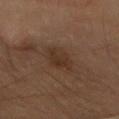Findings:
- notes — imaged on a skin check; not biopsied
- diameter — about 3 mm
- patient — female, aged around 55
- location — the head or neck
- image — ~15 mm crop, total-body skin-cancer survey
- illumination — cross-polarized